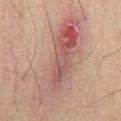Q: Was this lesion biopsied?
A: catalogued during a skin exam; not biopsied
Q: Lesion location?
A: the lower back
Q: How was the tile lit?
A: cross-polarized illumination
Q: What did automated image analysis measure?
A: an area of roughly 35 mm², an eccentricity of roughly 0.95, and two-axis asymmetry of about 0.3; border irregularity of about 5 on a 0–10 scale, a color-variation rating of about 10/10, and radial color variation of about 4.5; a classifier nevus-likeness of about 0/100
Q: Patient demographics?
A: male, aged 48 to 52
Q: What kind of image is this?
A: ~15 mm crop, total-body skin-cancer survey
Q: What is the lesion's diameter?
A: about 11 mm